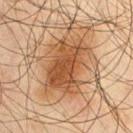<case>
<image>
  <source>total-body photography crop</source>
  <field_of_view_mm>15</field_of_view_mm>
</image>
<site>front of the torso</site>
<lesion_size>
  <long_diameter_mm_approx>7.0</long_diameter_mm_approx>
</lesion_size>
<patient>
  <sex>male</sex>
  <age_approx>45</age_approx>
</patient>
</case>